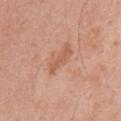Assessment: Part of a total-body skin-imaging series; this lesion was reviewed on a skin check and was not flagged for biopsy. Background: Longest diameter approximately 4 mm. The lesion is located on the front of the torso. The subject is a male about 70 years old. This is a white-light tile. Automated image analysis of the tile measured an average lesion color of about L≈58 a*≈24 b*≈34 (CIELAB). It also reported a border-irregularity index near 6.5/10, a color-variation rating of about 0/10, and a peripheral color-asymmetry measure near 0. A 15 mm close-up extracted from a 3D total-body photography capture.